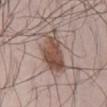Case summary:
– biopsy status — imaged on a skin check; not biopsied
– lesion size — about 6.5 mm
– image — ~15 mm tile from a whole-body skin photo
– anatomic site — the lower back
– tile lighting — white-light
– patient — male, aged around 40